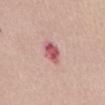image = ~15 mm crop, total-body skin-cancer survey
lighting = white-light
patient = female, about 60 years old
lesion diameter = about 3 mm
location = the abdomen
automated lesion analysis = a lesion color around L≈56 a*≈31 b*≈21 in CIELAB, roughly 14 lightness units darker than nearby skin, and a normalized lesion–skin contrast near 9; border irregularity of about 2 on a 0–10 scale and radial color variation of about 1.5; an automated nevus-likeness rating near 5 out of 100 and a lesion-detection confidence of about 100/100